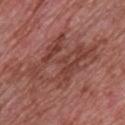Notes:
– illumination — white-light
– anatomic site — the chest
– subject — male, about 50 years old
– image-analysis metrics — a lesion area of about 33 mm², a shape eccentricity near 0.8, and two-axis asymmetry of about 0.35; a border-irregularity index near 6/10; an automated nevus-likeness rating near 0 out of 100 and a lesion-detection confidence of about 95/100
– image — ~15 mm crop, total-body skin-cancer survey